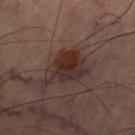The lesion was photographed on a routine skin check and not biopsied; there is no pathology result. Measured at roughly 6 mm in maximum diameter. A region of skin cropped from a whole-body photographic capture, roughly 15 mm wide. Imaged with cross-polarized lighting. From the right thigh.